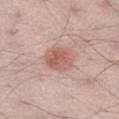Captured during whole-body skin photography for melanoma surveillance; the lesion was not biopsied. A male patient, in their 40s. Longest diameter approximately 3.5 mm. The total-body-photography lesion software estimated a lesion–skin lightness drop of about 10 and a normalized border contrast of about 7. It also reported a border-irregularity index near 1.5/10, a within-lesion color-variation index near 4/10, and a peripheral color-asymmetry measure near 1.5. The lesion is on the left thigh. Cropped from a whole-body photographic skin survey; the tile spans about 15 mm.